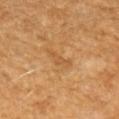  biopsy_status: not biopsied; imaged during a skin examination
  image:
    source: total-body photography crop
    field_of_view_mm: 15
  patient:
    sex: female
    age_approx: 50
  site: arm